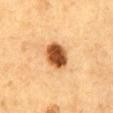| field | value |
|---|---|
| workup | total-body-photography surveillance lesion; no biopsy |
| subject | male, in their mid- to late 80s or older |
| acquisition | ~15 mm tile from a whole-body skin photo |
| lesion size | ≈3.5 mm |
| body site | the abdomen |
| illumination | cross-polarized |